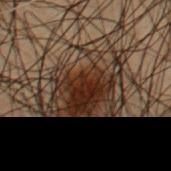Recorded during total-body skin imaging; not selected for excision or biopsy. The lesion is located on the chest. This is a cross-polarized tile. A 15 mm crop from a total-body photograph taken for skin-cancer surveillance. A male patient aged 53–57.